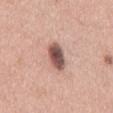| feature | finding |
|---|---|
| notes | total-body-photography surveillance lesion; no biopsy |
| lesion size | about 4.5 mm |
| patient | male, about 60 years old |
| tile lighting | white-light illumination |
| image source | 15 mm crop, total-body photography |
| site | the mid back |